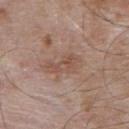Assessment:
The lesion was tiled from a total-body skin photograph and was not biopsied.
Image and clinical context:
Measured at roughly 4 mm in maximum diameter. Imaged with white-light lighting. A close-up tile cropped from a whole-body skin photograph, about 15 mm across. On the upper back. A male subject, roughly 55 years of age.